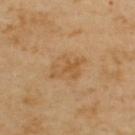Case summary:
• notes: total-body-photography surveillance lesion; no biopsy
• diameter: about 4 mm
• lighting: cross-polarized illumination
• patient: male, in their mid- to late 50s
• image source: total-body-photography crop, ~15 mm field of view
• location: the upper back